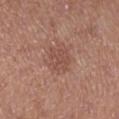automated metrics = a normalized border contrast of about 5; an automated nevus-likeness rating near 0 out of 100 | anatomic site = the right lower leg | lesion size = ≈3.5 mm | image source = ~15 mm crop, total-body skin-cancer survey | patient = female, approximately 40 years of age.